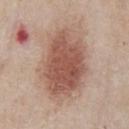Clinical impression:
Recorded during total-body skin imaging; not selected for excision or biopsy.
Background:
This image is a 15 mm lesion crop taken from a total-body photograph. An algorithmic analysis of the crop reported a mean CIELAB color near L≈54 a*≈21 b*≈27, about 13 CIELAB-L* units darker than the surrounding skin, and a normalized lesion–skin contrast near 9. It also reported a border-irregularity index near 2.5/10, internal color variation of about 4 on a 0–10 scale, and peripheral color asymmetry of about 1. The analysis additionally found an automated nevus-likeness rating near 100 out of 100 and a lesion-detection confidence of about 100/100. The patient is a male aged around 60. From the chest.